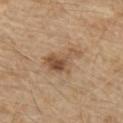workup: imaged on a skin check; not biopsied
patient: male, aged approximately 70
anatomic site: the chest
imaging modality: 15 mm crop, total-body photography
lighting: white-light illumination